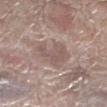image: 15 mm crop, total-body photography
body site: the left lower leg
lesion size: ~3.5 mm (longest diameter)
tile lighting: white-light illumination
image-analysis metrics: a lesion area of about 7 mm², an outline eccentricity of about 0.7 (0 = round, 1 = elongated), and a symmetry-axis asymmetry near 0.55; lesion-presence confidence of about 65/100
patient: male, aged 68 to 72